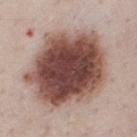- follow-up · total-body-photography surveillance lesion; no biopsy
- size · ~10 mm (longest diameter)
- location · the chest
- patient · male, aged 48–52
- image source · ~15 mm crop, total-body skin-cancer survey
- automated lesion analysis · a lesion area of about 60 mm² and a shape eccentricity near 0.5; a mean CIELAB color near L≈48 a*≈20 b*≈23 and a lesion–skin lightness drop of about 21; internal color variation of about 7.5 on a 0–10 scale and peripheral color asymmetry of about 2